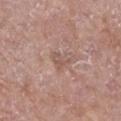follow-up: imaged on a skin check; not biopsied | lighting: white-light illumination | image-analysis metrics: a lesion area of about 3 mm² and two-axis asymmetry of about 0.5; an automated nevus-likeness rating near 0 out of 100 and a lesion-detection confidence of about 100/100 | patient: female, approximately 75 years of age | acquisition: total-body-photography crop, ~15 mm field of view | anatomic site: the right lower leg.